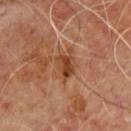Imaged during a routine full-body skin examination; the lesion was not biopsied and no histopathology is available.
About 3 mm across.
This is a cross-polarized tile.
A male subject, in their 60s.
On the back.
A roughly 15 mm field-of-view crop from a total-body skin photograph.